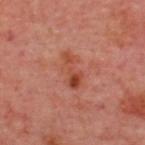Assessment:
Captured during whole-body skin photography for melanoma surveillance; the lesion was not biopsied.
Clinical summary:
The patient is approximately 55 years of age. Located on the upper back. A 15 mm crop from a total-body photograph taken for skin-cancer surveillance.